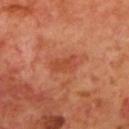Assessment:
Captured during whole-body skin photography for melanoma surveillance; the lesion was not biopsied.
Background:
Located on the mid back. Automated image analysis of the tile measured a lesion area of about 5 mm², a shape eccentricity near 0.85, and a symmetry-axis asymmetry near 0.3. The analysis additionally found lesion-presence confidence of about 100/100. A male patient, roughly 70 years of age. Approximately 3 mm at its widest. Captured under cross-polarized illumination. Cropped from a total-body skin-imaging series; the visible field is about 15 mm.